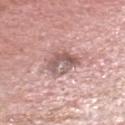The lesion was tiled from a total-body skin photograph and was not biopsied.
The lesion's longest dimension is about 3.5 mm.
Located on the head or neck.
The tile uses white-light illumination.
This image is a 15 mm lesion crop taken from a total-body photograph.
A male patient, about 70 years old.